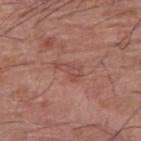Imaged during a routine full-body skin examination; the lesion was not biopsied and no histopathology is available. A region of skin cropped from a whole-body photographic capture, roughly 15 mm wide. This is a white-light tile. A male subject aged 53 to 57. On the left upper arm. The total-body-photography lesion software estimated an area of roughly 4 mm², an eccentricity of roughly 0.9, and a symmetry-axis asymmetry near 0.5. And it measured a mean CIELAB color near L≈48 a*≈24 b*≈26, a lesion–skin lightness drop of about 7, and a lesion-to-skin contrast of about 5 (normalized; higher = more distinct). About 3.5 mm across.